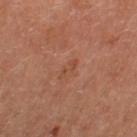notes = imaged on a skin check; not biopsied | lesion size = ≈2.5 mm | anatomic site = the left thigh | patient = male, about 65 years old | automated lesion analysis = a lesion area of about 2.5 mm² and a symmetry-axis asymmetry near 0.35; roughly 5 lightness units darker than nearby skin and a normalized lesion–skin contrast near 4.5; border irregularity of about 4 on a 0–10 scale and a peripheral color-asymmetry measure near 0; lesion-presence confidence of about 100/100 | imaging modality = ~15 mm crop, total-body skin-cancer survey.